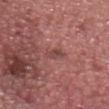notes — total-body-photography surveillance lesion; no biopsy